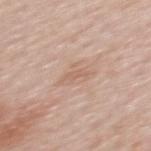The lesion was tiled from a total-body skin photograph and was not biopsied.
Captured under white-light illumination.
A 15 mm close-up tile from a total-body photography series done for melanoma screening.
The lesion is located on the back.
The subject is a female aged 58 to 62.